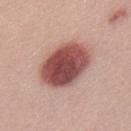Findings:
* acquisition · 15 mm crop, total-body photography
* tile lighting · white-light illumination
* diameter · ≈6.5 mm
* subject · male, approximately 30 years of age